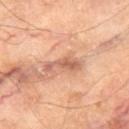No biopsy was performed on this lesion — it was imaged during a full skin examination and was not determined to be concerning. About 4.5 mm across. A roughly 15 mm field-of-view crop from a total-body skin photograph. The tile uses cross-polarized illumination. The subject is a male about 70 years old. The lesion is on the right thigh.